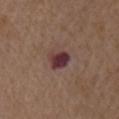The patient is a male aged 73–77. A lesion tile, about 15 mm wide, cut from a 3D total-body photograph. From the right upper arm.